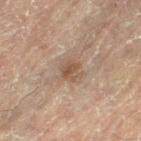anatomic site — the right leg | image source — ~15 mm tile from a whole-body skin photo | lesion diameter — about 2.5 mm | patient — female, about 80 years old.